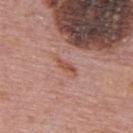Recorded during total-body skin imaging; not selected for excision or biopsy. Imaged with white-light lighting. Cropped from a whole-body photographic skin survey; the tile spans about 15 mm. A male subject in their mid- to late 70s. An algorithmic analysis of the crop reported a lesion area of about 2.5 mm² and two-axis asymmetry of about 0.4. The analysis additionally found a normalized border contrast of about 7.5. The analysis additionally found border irregularity of about 4 on a 0–10 scale, a within-lesion color-variation index near 0/10, and peripheral color asymmetry of about 0. On the upper back. Longest diameter approximately 2.5 mm.